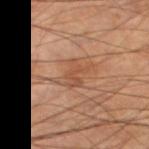The lesion is located on the left thigh.
Cropped from a whole-body photographic skin survey; the tile spans about 15 mm.
A male subject in their 60s.